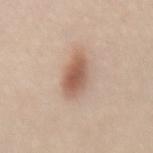{"biopsy_status": "not biopsied; imaged during a skin examination", "lighting": "white-light", "site": "lower back", "image": {"source": "total-body photography crop", "field_of_view_mm": 15}, "patient": {"sex": "female", "age_approx": 20}}